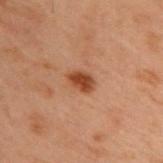Part of a total-body skin-imaging series; this lesion was reviewed on a skin check and was not flagged for biopsy. The tile uses cross-polarized illumination. Located on the upper back. A lesion tile, about 15 mm wide, cut from a 3D total-body photograph. The subject is a male aged 53 to 57. Approximately 2.5 mm at its widest.